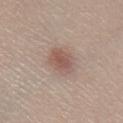- imaging modality — ~15 mm crop, total-body skin-cancer survey
- image-analysis metrics — a footprint of about 8 mm², an outline eccentricity of about 0.7 (0 = round, 1 = elongated), and a symmetry-axis asymmetry near 0.2; an average lesion color of about L≈54 a*≈18 b*≈23 (CIELAB), roughly 10 lightness units darker than nearby skin, and a lesion-to-skin contrast of about 6.5 (normalized; higher = more distinct)
- patient — female, approximately 25 years of age
- site — the right lower leg
- diameter — ~4 mm (longest diameter)